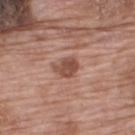Imaged during a routine full-body skin examination; the lesion was not biopsied and no histopathology is available.